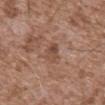biopsy status: catalogued during a skin exam; not biopsied | patient: male, in their mid-70s | image: ~15 mm tile from a whole-body skin photo | size: about 3 mm | illumination: white-light | anatomic site: the front of the torso.